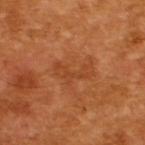Captured during whole-body skin photography for melanoma surveillance; the lesion was not biopsied. Approximately 4.5 mm at its widest. The tile uses cross-polarized illumination. Cropped from a whole-body photographic skin survey; the tile spans about 15 mm. The total-body-photography lesion software estimated an eccentricity of roughly 0.9 and two-axis asymmetry of about 0.55. It also reported an average lesion color of about L≈43 a*≈27 b*≈38 (CIELAB), a lesion–skin lightness drop of about 6, and a lesion-to-skin contrast of about 5 (normalized; higher = more distinct). And it measured peripheral color asymmetry of about 0.5. The analysis additionally found a classifier nevus-likeness of about 0/100 and a lesion-detection confidence of about 100/100. The patient is a male in their mid-60s.